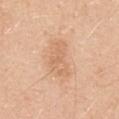The lesion was tiled from a total-body skin photograph and was not biopsied. Longest diameter approximately 4.5 mm. A close-up tile cropped from a whole-body skin photograph, about 15 mm across. Imaged with white-light lighting. On the abdomen. A male patient, roughly 50 years of age. The lesion-visualizer software estimated a symmetry-axis asymmetry near 0.35. And it measured a mean CIELAB color near L≈67 a*≈21 b*≈35, roughly 7 lightness units darker than nearby skin, and a lesion-to-skin contrast of about 4.5 (normalized; higher = more distinct).